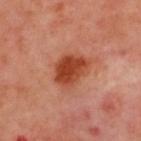Impression: Part of a total-body skin-imaging series; this lesion was reviewed on a skin check and was not flagged for biopsy. Image and clinical context: A close-up tile cropped from a whole-body skin photograph, about 15 mm across. The recorded lesion diameter is about 4.5 mm. On the upper back. Automated tile analysis of the lesion measured a footprint of about 12 mm², an outline eccentricity of about 0.65 (0 = round, 1 = elongated), and two-axis asymmetry of about 0.2. The analysis additionally found border irregularity of about 2 on a 0–10 scale, a within-lesion color-variation index near 4.5/10, and a peripheral color-asymmetry measure near 1.5. The software also gave a nevus-likeness score of about 100/100 and a lesion-detection confidence of about 100/100. A male subject, roughly 50 years of age. Captured under cross-polarized illumination.